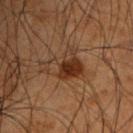Case summary:
• subject: male, aged approximately 50
• lighting: cross-polarized
• acquisition: total-body-photography crop, ~15 mm field of view
• lesion size: about 4.5 mm
• body site: the left upper arm
• automated lesion analysis: roughly 7 lightness units darker than nearby skin; internal color variation of about 8 on a 0–10 scale